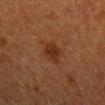The lesion was tiled from a total-body skin photograph and was not biopsied. The total-body-photography lesion software estimated a lesion area of about 5 mm². And it measured a nevus-likeness score of about 60/100. This is a cross-polarized tile. On the right forearm. A female patient, aged 38 to 42. Approximately 3 mm at its widest. A region of skin cropped from a whole-body photographic capture, roughly 15 mm wide.